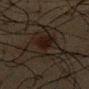Part of a total-body skin-imaging series; this lesion was reviewed on a skin check and was not flagged for biopsy.
A male subject, in their 60s.
Cropped from a whole-body photographic skin survey; the tile spans about 15 mm.
An algorithmic analysis of the crop reported a shape eccentricity near 0.8 and two-axis asymmetry of about 0.45.
The lesion is on the chest.
Captured under cross-polarized illumination.